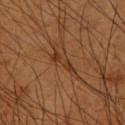| field | value |
|---|---|
| notes | imaged on a skin check; not biopsied |
| imaging modality | ~15 mm crop, total-body skin-cancer survey |
| site | the right upper arm |
| subject | male, approximately 60 years of age |